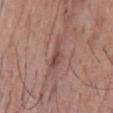Clinical impression: No biopsy was performed on this lesion — it was imaged during a full skin examination and was not determined to be concerning. Image and clinical context: From the chest. A 15 mm crop from a total-body photograph taken for skin-cancer surveillance. Captured under white-light illumination. Automated image analysis of the tile measured a footprint of about 5.5 mm², a shape eccentricity near 0.95, and two-axis asymmetry of about 0.3. A male subject aged approximately 55. About 5 mm across.